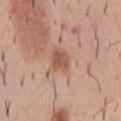The lesion was photographed on a routine skin check and not biopsied; there is no pathology result. Captured under white-light illumination. Longest diameter approximately 3 mm. From the front of the torso. The patient is a male aged 38 to 42. Cropped from a total-body skin-imaging series; the visible field is about 15 mm.